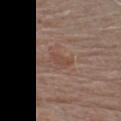<tbp_lesion>
  <biopsy_status>not biopsied; imaged during a skin examination</biopsy_status>
  <lesion_size>
    <long_diameter_mm_approx>2.5</long_diameter_mm_approx>
  </lesion_size>
  <lighting>white-light</lighting>
  <site>chest</site>
  <image>
    <source>total-body photography crop</source>
    <field_of_view_mm>15</field_of_view_mm>
  </image>
  <patient>
    <sex>male</sex>
    <age_approx>75</age_approx>
  </patient>
</tbp_lesion>